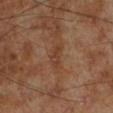Recorded during total-body skin imaging; not selected for excision or biopsy.
This is a cross-polarized tile.
The subject is a male aged 68 to 72.
The lesion is on the left lower leg.
A close-up tile cropped from a whole-body skin photograph, about 15 mm across.
Automated image analysis of the tile measured a lesion area of about 4 mm², an eccentricity of roughly 0.9, and a symmetry-axis asymmetry near 0.35. And it measured a lesion color around L≈38 a*≈21 b*≈29 in CIELAB, a lesion–skin lightness drop of about 6, and a normalized border contrast of about 5.5.